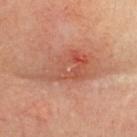Case summary:
- follow-up — total-body-photography surveillance lesion; no biopsy
- subject — female, in their mid- to late 70s
- illumination — cross-polarized
- automated lesion analysis — a footprint of about 17 mm², an outline eccentricity of about 0.85 (0 = round, 1 = elongated), and a shape-asymmetry score of about 0.4 (0 = symmetric); a normalized border contrast of about 6
- diameter — ~7.5 mm (longest diameter)
- acquisition — total-body-photography crop, ~15 mm field of view
- location — the head or neck